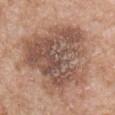Impression:
The lesion was photographed on a routine skin check and not biopsied; there is no pathology result.
Acquisition and patient details:
Measured at roughly 9.5 mm in maximum diameter. From the right upper arm. A male subject aged 58 to 62. Cropped from a whole-body photographic skin survey; the tile spans about 15 mm. The total-body-photography lesion software estimated a lesion area of about 60 mm² and an eccentricity of roughly 0.4. And it measured a border-irregularity index near 4/10, internal color variation of about 7.5 on a 0–10 scale, and peripheral color asymmetry of about 2.5. The analysis additionally found a nevus-likeness score of about 35/100. The tile uses white-light illumination.